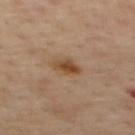Acquisition and patient details: A close-up tile cropped from a whole-body skin photograph, about 15 mm across. From the mid back. A male patient, in their mid-50s. About 2.5 mm across. This is a cross-polarized tile.